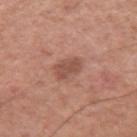  biopsy_status: not biopsied; imaged during a skin examination
  patient:
    sex: male
    age_approx: 55
  site: left upper arm
  lesion_size:
    long_diameter_mm_approx: 3.0
  lighting: white-light
  image:
    source: total-body photography crop
    field_of_view_mm: 15